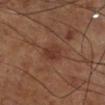Case summary:
- workup — no biopsy performed (imaged during a skin exam)
- subject — male, in their 70s
- acquisition — total-body-photography crop, ~15 mm field of view
- location — the right lower leg
- lighting — cross-polarized